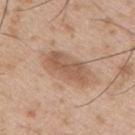Automated image analysis of the tile measured border irregularity of about 2 on a 0–10 scale, a color-variation rating of about 3/10, and radial color variation of about 1. And it measured a classifier nevus-likeness of about 25/100 and lesion-presence confidence of about 100/100. The lesion is located on the right upper arm. Measured at roughly 5.5 mm in maximum diameter. Cropped from a total-body skin-imaging series; the visible field is about 15 mm. A male patient, aged 53–57.